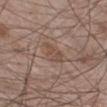Assessment:
Part of a total-body skin-imaging series; this lesion was reviewed on a skin check and was not flagged for biopsy.
Clinical summary:
A male subject, aged 58–62. Located on the right lower leg. The tile uses white-light illumination. A roughly 15 mm field-of-view crop from a total-body skin photograph. Measured at roughly 3.5 mm in maximum diameter. The lesion-visualizer software estimated a footprint of about 5.5 mm² and a shape-asymmetry score of about 0.3 (0 = symmetric). The software also gave a classifier nevus-likeness of about 0/100 and lesion-presence confidence of about 100/100.